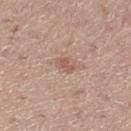workup: imaged on a skin check; not biopsied | anatomic site: the right lower leg | patient: female, aged approximately 45 | image: 15 mm crop, total-body photography | diameter: ≈2.5 mm | illumination: white-light illumination | automated metrics: a lesion area of about 3 mm² and a shape eccentricity near 0.8; border irregularity of about 4 on a 0–10 scale, internal color variation of about 0 on a 0–10 scale, and peripheral color asymmetry of about 0; a classifier nevus-likeness of about 0/100 and a lesion-detection confidence of about 100/100.